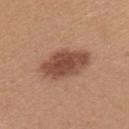workup: catalogued during a skin exam; not biopsied
body site: the left upper arm
subject: female, aged approximately 45
image source: 15 mm crop, total-body photography
automated lesion analysis: a lesion–skin lightness drop of about 13 and a normalized lesion–skin contrast near 9.5; a border-irregularity index near 2/10, a within-lesion color-variation index near 3.5/10, and radial color variation of about 1; a nevus-likeness score of about 95/100 and a lesion-detection confidence of about 100/100